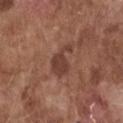No biopsy was performed on this lesion — it was imaged during a full skin examination and was not determined to be concerning. From the front of the torso. This is a white-light tile. Approximately 4 mm at its widest. The lesion-visualizer software estimated a lesion area of about 7 mm². It also reported a mean CIELAB color near L≈40 a*≈21 b*≈25, roughly 9 lightness units darker than nearby skin, and a lesion-to-skin contrast of about 7.5 (normalized; higher = more distinct). The analysis additionally found a border-irregularity index near 5.5/10 and radial color variation of about 0.5. A male patient, aged around 75. Cropped from a total-body skin-imaging series; the visible field is about 15 mm.